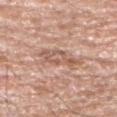workup: no biopsy performed (imaged during a skin exam); lighting: white-light illumination; subject: male, in their 80s; size: about 4.5 mm; body site: the arm; image source: 15 mm crop, total-body photography.